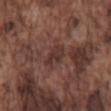Impression: No biopsy was performed on this lesion — it was imaged during a full skin examination and was not determined to be concerning. Background: The tile uses white-light illumination. A male subject roughly 75 years of age. A lesion tile, about 15 mm wide, cut from a 3D total-body photograph. About 3 mm across. The lesion is located on the back.